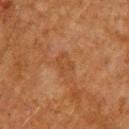workup: imaged on a skin check; not biopsied
site: the upper back
lesion diameter: ~3 mm (longest diameter)
tile lighting: cross-polarized illumination
image: 15 mm crop, total-body photography
automated metrics: a footprint of about 4 mm², an outline eccentricity of about 0.7 (0 = round, 1 = elongated), and two-axis asymmetry of about 0.3; a classifier nevus-likeness of about 0/100 and lesion-presence confidence of about 100/100
patient: male, roughly 65 years of age